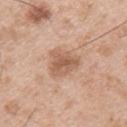No biopsy was performed on this lesion — it was imaged during a full skin examination and was not determined to be concerning. The lesion is located on the left upper arm. Cropped from a whole-body photographic skin survey; the tile spans about 15 mm. The subject is a male aged 53–57.